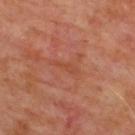Image and clinical context:
The subject is a male about 65 years old. A lesion tile, about 15 mm wide, cut from a 3D total-body photograph. Automated image analysis of the tile measured a lesion color around L≈45 a*≈27 b*≈33 in CIELAB and roughly 5 lightness units darker than nearby skin. And it measured an automated nevus-likeness rating near 0 out of 100. The lesion is located on the upper back. Captured under cross-polarized illumination. Measured at roughly 3 mm in maximum diameter.